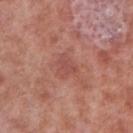notes — catalogued during a skin exam; not biopsied
automated metrics — an average lesion color of about L≈50 a*≈26 b*≈27 (CIELAB), a lesion–skin lightness drop of about 6, and a lesion-to-skin contrast of about 4.5 (normalized; higher = more distinct)
imaging modality — ~15 mm crop, total-body skin-cancer survey
body site — the right lower leg
subject — male, aged 53–57
lesion diameter — ~2.5 mm (longest diameter)